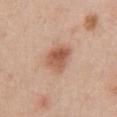  biopsy_status: not biopsied; imaged during a skin examination
  automated_metrics:
    cielab_L: 57
    cielab_a: 22
    cielab_b: 31
    vs_skin_darker_L: 12.0
    vs_skin_contrast_norm: 8.0
    nevus_likeness_0_100: 95
    lesion_detection_confidence_0_100: 100
  site: chest
  image:
    source: total-body photography crop
    field_of_view_mm: 15
  patient:
    sex: male
    age_approx: 55